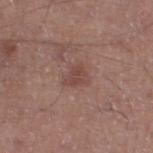{
  "biopsy_status": "not biopsied; imaged during a skin examination",
  "patient": {
    "sex": "male",
    "age_approx": 55
  },
  "image": {
    "source": "total-body photography crop",
    "field_of_view_mm": 15
  },
  "site": "leg"
}